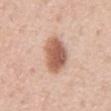  biopsy_status: not biopsied; imaged during a skin examination
  site: abdomen
  image:
    source: total-body photography crop
    field_of_view_mm: 15
  automated_metrics:
    color_variation_0_10: 4.5
    peripheral_color_asymmetry: 1.5
    nevus_likeness_0_100: 100
    lesion_detection_confidence_0_100: 100
  lesion_size:
    long_diameter_mm_approx: 5.5
  patient:
    sex: male
    age_approx: 55
  lighting: white-light